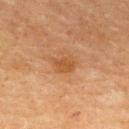Impression:
Recorded during total-body skin imaging; not selected for excision or biopsy.
Clinical summary:
From the upper back. A female subject, in their mid-50s. A region of skin cropped from a whole-body photographic capture, roughly 15 mm wide.